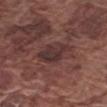Case summary:
* biopsy status · imaged on a skin check; not biopsied
* lighting · white-light
* automated lesion analysis · a lesion color around L≈33 a*≈18 b*≈18 in CIELAB, roughly 7 lightness units darker than nearby skin, and a normalized border contrast of about 8; a border-irregularity index near 2/10, internal color variation of about 4 on a 0–10 scale, and a peripheral color-asymmetry measure near 1.5; an automated nevus-likeness rating near 5 out of 100 and a detector confidence of about 90 out of 100 that the crop contains a lesion
* image · 15 mm crop, total-body photography
* subject · male, about 75 years old
* body site · the right upper arm
* diameter · about 5 mm